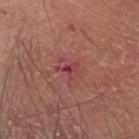Part of a total-body skin-imaging series; this lesion was reviewed on a skin check and was not flagged for biopsy.
A close-up tile cropped from a whole-body skin photograph, about 15 mm across.
The lesion's longest dimension is about 2.5 mm.
The patient is a male approximately 65 years of age.
The tile uses white-light illumination.
From the head or neck.